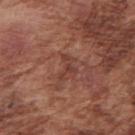Clinical summary: Cropped from a whole-body photographic skin survey; the tile spans about 15 mm. Automated image analysis of the tile measured roughly 7 lightness units darker than nearby skin and a normalized lesion–skin contrast near 6. It also reported a nevus-likeness score of about 0/100 and a lesion-detection confidence of about 85/100. Imaged with white-light lighting. A male patient, in their mid- to late 70s. On the right upper arm.